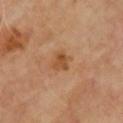The lesion was photographed on a routine skin check and not biopsied; there is no pathology result.
This is a cross-polarized tile.
The lesion is on the front of the torso.
A close-up tile cropped from a whole-body skin photograph, about 15 mm across.
The subject is a male roughly 65 years of age.
The lesion's longest dimension is about 2.5 mm.